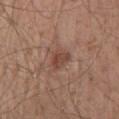• anatomic site: the mid back
• patient: male, aged 53–57
• illumination: white-light
• imaging modality: 15 mm crop, total-body photography
• diameter: ~3 mm (longest diameter)
• automated metrics: a footprint of about 5 mm², a shape eccentricity near 0.65, and a symmetry-axis asymmetry near 0.25; a lesion color around L≈45 a*≈21 b*≈26 in CIELAB, roughly 8 lightness units darker than nearby skin, and a normalized lesion–skin contrast near 6.5; a lesion-detection confidence of about 100/100